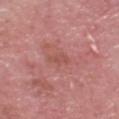notes = total-body-photography surveillance lesion; no biopsy
TBP lesion metrics = an area of roughly 2.5 mm², an eccentricity of roughly 0.95, and two-axis asymmetry of about 0.4; a color-variation rating of about 0/10
imaging modality = ~15 mm tile from a whole-body skin photo
illumination = white-light
subject = male, aged 63–67
body site = the head or neck